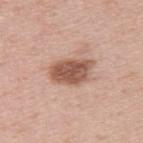Case summary:
• follow-up: total-body-photography surveillance lesion; no biopsy
• diameter: ≈5 mm
• subject: male, aged around 50
• anatomic site: the upper back
• image: 15 mm crop, total-body photography